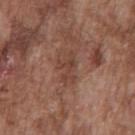Background:
The subject is a male aged 73 to 77. A 15 mm crop from a total-body photograph taken for skin-cancer surveillance. The lesion-visualizer software estimated a footprint of about 7 mm², an eccentricity of roughly 0.85, and two-axis asymmetry of about 0.35. It also reported a lesion color around L≈42 a*≈21 b*≈26 in CIELAB and a normalized border contrast of about 6. The software also gave a border-irregularity rating of about 4.5/10, a within-lesion color-variation index near 2.5/10, and a peripheral color-asymmetry measure near 0.5. This is a white-light tile. Longest diameter approximately 4 mm. The lesion is on the chest.